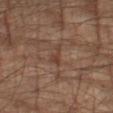Q: Is there a histopathology result?
A: total-body-photography surveillance lesion; no biopsy
Q: Illumination type?
A: cross-polarized
Q: Lesion size?
A: about 3 mm
Q: What is the anatomic site?
A: the left forearm
Q: What are the patient's age and sex?
A: male, aged approximately 65
Q: What is the imaging modality?
A: 15 mm crop, total-body photography